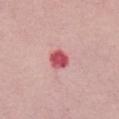  biopsy_status: not biopsied; imaged during a skin examination
  lesion_size:
    long_diameter_mm_approx: 2.5
  site: chest
  patient:
    sex: female
    age_approx: 60
  image:
    source: total-body photography crop
    field_of_view_mm: 15